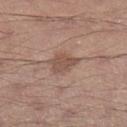Findings:
* notes · catalogued during a skin exam; not biopsied
* subject · male, aged approximately 30
* image · total-body-photography crop, ~15 mm field of view
* TBP lesion metrics · a shape-asymmetry score of about 0.25 (0 = symmetric); a mean CIELAB color near L≈51 a*≈19 b*≈25, about 9 CIELAB-L* units darker than the surrounding skin, and a lesion-to-skin contrast of about 6.5 (normalized; higher = more distinct); a lesion-detection confidence of about 100/100
* location · the left lower leg
* size · ~3.5 mm (longest diameter)
* tile lighting · white-light